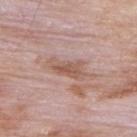Image and clinical context: The recorded lesion diameter is about 4 mm. The total-body-photography lesion software estimated a border-irregularity index near 4/10, a within-lesion color-variation index near 3/10, and radial color variation of about 1. Cropped from a whole-body photographic skin survey; the tile spans about 15 mm. This is a white-light tile. A male patient, in their 60s. The lesion is on the back.